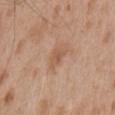Clinical impression: Recorded during total-body skin imaging; not selected for excision or biopsy. Background: A region of skin cropped from a whole-body photographic capture, roughly 15 mm wide. The lesion is located on the chest. A male subject, roughly 65 years of age. The tile uses white-light illumination. Longest diameter approximately 3 mm.